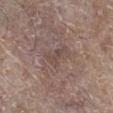Impression:
Captured during whole-body skin photography for melanoma surveillance; the lesion was not biopsied.
Acquisition and patient details:
A male patient aged 63 to 67. The total-body-photography lesion software estimated a mean CIELAB color near L≈46 a*≈16 b*≈20, a lesion–skin lightness drop of about 6, and a normalized border contrast of about 5. The analysis additionally found a border-irregularity index near 7/10. Measured at roughly 3.5 mm in maximum diameter. From the right lower leg. This is a white-light tile. A 15 mm close-up extracted from a 3D total-body photography capture.